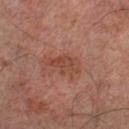Captured during whole-body skin photography for melanoma surveillance; the lesion was not biopsied.
Located on the left thigh.
Imaged with cross-polarized lighting.
The recorded lesion diameter is about 4 mm.
A male patient roughly 65 years of age.
An algorithmic analysis of the crop reported a border-irregularity rating of about 4/10 and radial color variation of about 1. And it measured an automated nevus-likeness rating near 0 out of 100 and a detector confidence of about 100 out of 100 that the crop contains a lesion.
A 15 mm crop from a total-body photograph taken for skin-cancer surveillance.